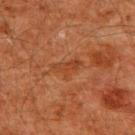tile lighting = cross-polarized | subject = male, roughly 60 years of age | body site = the upper back | image source = total-body-photography crop, ~15 mm field of view.